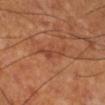Q: Was this lesion biopsied?
A: imaged on a skin check; not biopsied
Q: What did automated image analysis measure?
A: a footprint of about 5 mm² and a symmetry-axis asymmetry near 0.55; a border-irregularity rating of about 7.5/10, a within-lesion color-variation index near 2/10, and peripheral color asymmetry of about 0.5; an automated nevus-likeness rating near 0 out of 100
Q: What is the lesion's diameter?
A: about 4 mm
Q: What is the imaging modality?
A: total-body-photography crop, ~15 mm field of view
Q: Where on the body is the lesion?
A: the right lower leg
Q: What are the patient's age and sex?
A: male, roughly 70 years of age